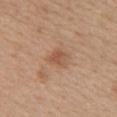Clinical impression:
This lesion was catalogued during total-body skin photography and was not selected for biopsy.
Acquisition and patient details:
The lesion's longest dimension is about 2.5 mm. Imaged with white-light lighting. From the upper back. Cropped from a whole-body photographic skin survey; the tile spans about 15 mm. A female patient in their mid-20s.